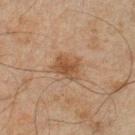Notes:
* follow-up: total-body-photography surveillance lesion; no biopsy